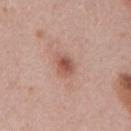Clinical impression: The lesion was tiled from a total-body skin photograph and was not biopsied. Image and clinical context: The lesion's longest dimension is about 3 mm. Automated image analysis of the tile measured an eccentricity of roughly 0.75 and a shape-asymmetry score of about 0.25 (0 = symmetric). It also reported an average lesion color of about L≈54 a*≈23 b*≈27 (CIELAB) and a lesion-to-skin contrast of about 7.5 (normalized; higher = more distinct). And it measured border irregularity of about 2.5 on a 0–10 scale, a color-variation rating of about 5.5/10, and peripheral color asymmetry of about 1.5. The analysis additionally found an automated nevus-likeness rating near 60 out of 100 and a lesion-detection confidence of about 100/100. This is a white-light tile. The subject is a male about 55 years old. A 15 mm close-up tile from a total-body photography series done for melanoma screening. On the mid back.